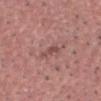biopsy_status: not biopsied; imaged during a skin examination
site: head or neck
patient:
  sex: male
  age_approx: 55
image:
  source: total-body photography crop
  field_of_view_mm: 15